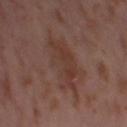biopsy status: catalogued during a skin exam; not biopsied
acquisition: 15 mm crop, total-body photography
subject: female, approximately 55 years of age
site: the left thigh
size: about 8 mm
automated lesion analysis: an area of roughly 21 mm², a shape eccentricity near 0.85, and a shape-asymmetry score of about 0.35 (0 = symmetric); a border-irregularity rating of about 5.5/10; a nevus-likeness score of about 0/100 and a detector confidence of about 100 out of 100 that the crop contains a lesion